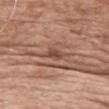Recorded during total-body skin imaging; not selected for excision or biopsy.
Located on the chest.
The lesion-visualizer software estimated a mean CIELAB color near L≈48 a*≈23 b*≈29 and about 9 CIELAB-L* units darker than the surrounding skin. It also reported a nevus-likeness score of about 5/100.
About 3.5 mm across.
Cropped from a total-body skin-imaging series; the visible field is about 15 mm.
The subject is a female aged around 65.